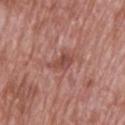Impression:
No biopsy was performed on this lesion — it was imaged during a full skin examination and was not determined to be concerning.
Background:
A male subject, aged 68–72. On the upper back. The tile uses white-light illumination. A 15 mm crop from a total-body photograph taken for skin-cancer surveillance. Automated tile analysis of the lesion measured an automated nevus-likeness rating near 0 out of 100. Measured at roughly 3.5 mm in maximum diameter.